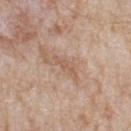workup — catalogued during a skin exam; not biopsied | automated metrics — a lesion area of about 2 mm², an eccentricity of roughly 0.95, and two-axis asymmetry of about 0.25; a mean CIELAB color near L≈57 a*≈19 b*≈29, roughly 7 lightness units darker than nearby skin, and a lesion-to-skin contrast of about 5 (normalized; higher = more distinct); a border-irregularity index near 3.5/10, a color-variation rating of about 0/10, and a peripheral color-asymmetry measure near 0; a detector confidence of about 90 out of 100 that the crop contains a lesion | imaging modality — ~15 mm crop, total-body skin-cancer survey | lighting — white-light illumination | size — ≈2.5 mm | location — the upper back | subject — male, aged around 80.